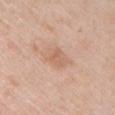follow-up=imaged on a skin check; not biopsied | body site=the front of the torso | TBP lesion metrics=border irregularity of about 2 on a 0–10 scale, a within-lesion color-variation index near 2.5/10, and a peripheral color-asymmetry measure near 1; an automated nevus-likeness rating near 5 out of 100 and lesion-presence confidence of about 100/100 | subject=female, roughly 65 years of age | illumination=white-light | image=total-body-photography crop, ~15 mm field of view.